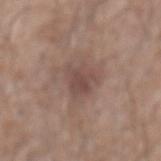| feature | finding |
|---|---|
| follow-up | total-body-photography surveillance lesion; no biopsy |
| acquisition | ~15 mm crop, total-body skin-cancer survey |
| lesion diameter | ~3.5 mm (longest diameter) |
| patient | male, approximately 60 years of age |
| location | the mid back |